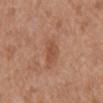Recorded during total-body skin imaging; not selected for excision or biopsy. A male patient, aged approximately 75. Located on the abdomen. A lesion tile, about 15 mm wide, cut from a 3D total-body photograph.